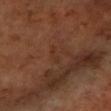Assessment: Captured during whole-body skin photography for melanoma surveillance; the lesion was not biopsied. Background: The tile uses cross-polarized illumination. About 3 mm across. The patient is a female about 70 years old. A region of skin cropped from a whole-body photographic capture, roughly 15 mm wide. Automated image analysis of the tile measured an area of roughly 2.5 mm² and two-axis asymmetry of about 0.45. The software also gave a lesion–skin lightness drop of about 4. On the left forearm.